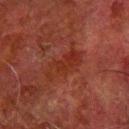No biopsy was performed on this lesion — it was imaged during a full skin examination and was not determined to be concerning.
The subject is a male roughly 80 years of age.
Automated image analysis of the tile measured a lesion color around L≈25 a*≈24 b*≈26 in CIELAB, a lesion–skin lightness drop of about 5, and a lesion-to-skin contrast of about 6 (normalized; higher = more distinct). And it measured a border-irregularity rating of about 4.5/10 and radial color variation of about 1.5. It also reported an automated nevus-likeness rating near 0 out of 100 and a lesion-detection confidence of about 100/100.
From the right forearm.
Captured under cross-polarized illumination.
A lesion tile, about 15 mm wide, cut from a 3D total-body photograph.